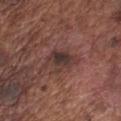Case summary:
- follow-up · total-body-photography surveillance lesion; no biopsy
- patient · male, aged approximately 75
- acquisition · ~15 mm crop, total-body skin-cancer survey
- anatomic site · the chest